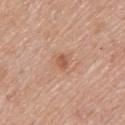Clinical impression: Imaged during a routine full-body skin examination; the lesion was not biopsied and no histopathology is available. Image and clinical context: On the upper back. The total-body-photography lesion software estimated a border-irregularity rating of about 3/10 and a color-variation rating of about 4/10. A female patient, about 65 years old. Measured at roughly 3 mm in maximum diameter. The tile uses white-light illumination. A 15 mm crop from a total-body photograph taken for skin-cancer surveillance.